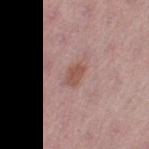Assessment: Captured during whole-body skin photography for melanoma surveillance; the lesion was not biopsied. Image and clinical context: A female patient, roughly 50 years of age. The lesion is located on the left thigh. The lesion's longest dimension is about 2.5 mm. A region of skin cropped from a whole-body photographic capture, roughly 15 mm wide. Captured under white-light illumination.